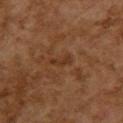– biopsy status: imaged on a skin check; not biopsied
– TBP lesion metrics: an outline eccentricity of about 0.95 (0 = round, 1 = elongated) and a shape-asymmetry score of about 0.4 (0 = symmetric)
– patient: female, aged approximately 60
– tile lighting: cross-polarized illumination
– lesion diameter: ~3 mm (longest diameter)
– location: the back
– image: ~15 mm tile from a whole-body skin photo